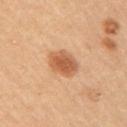Impression:
Part of a total-body skin-imaging series; this lesion was reviewed on a skin check and was not flagged for biopsy.
Background:
This is a cross-polarized tile. Longest diameter approximately 4.5 mm. A female subject, aged around 45. From the arm. Cropped from a whole-body photographic skin survey; the tile spans about 15 mm.